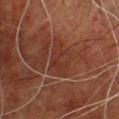workup — no biopsy performed (imaged during a skin exam) | site — the chest | patient — male, aged around 50 | imaging modality — total-body-photography crop, ~15 mm field of view.